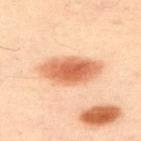The lesion was photographed on a routine skin check and not biopsied; there is no pathology result. Cropped from a total-body skin-imaging series; the visible field is about 15 mm. From the upper back. A male patient aged 48 to 52. Automated image analysis of the tile measured a footprint of about 17 mm², an outline eccentricity of about 0.9 (0 = round, 1 = elongated), and two-axis asymmetry of about 0.15.